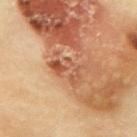{"biopsy_status": "not biopsied; imaged during a skin examination", "site": "upper back", "patient": {"sex": "female", "age_approx": 60}, "image": {"source": "total-body photography crop", "field_of_view_mm": 15}, "lighting": "cross-polarized", "automated_metrics": {"border_irregularity_0_10": 8.5, "color_variation_0_10": 7.5, "nevus_likeness_0_100": 0, "lesion_detection_confidence_0_100": 100}, "lesion_size": {"long_diameter_mm_approx": 4.5}}